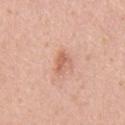| feature | finding |
|---|---|
| follow-up | imaged on a skin check; not biopsied |
| image source | 15 mm crop, total-body photography |
| diameter | ≈3 mm |
| automated metrics | an area of roughly 3.5 mm², an outline eccentricity of about 0.85 (0 = round, 1 = elongated), and two-axis asymmetry of about 0.45; an average lesion color of about L≈62 a*≈25 b*≈31 (CIELAB), a lesion–skin lightness drop of about 10, and a lesion-to-skin contrast of about 6.5 (normalized; higher = more distinct); a border-irregularity rating of about 4.5/10 and radial color variation of about 0.5; a nevus-likeness score of about 5/100 and a detector confidence of about 100 out of 100 that the crop contains a lesion |
| site | the abdomen |
| illumination | white-light |
| patient | male, about 55 years old |